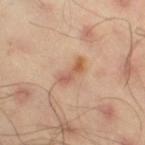patient: male, approximately 45 years of age | tile lighting: cross-polarized illumination | image: 15 mm crop, total-body photography | body site: the right thigh | automated metrics: a footprint of about 4 mm², an outline eccentricity of about 0.95 (0 = round, 1 = elongated), and a symmetry-axis asymmetry near 0.4; an average lesion color of about L≈57 a*≈22 b*≈32 (CIELAB) and about 10 CIELAB-L* units darker than the surrounding skin | lesion size: ≈3.5 mm.